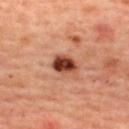Impression:
Captured during whole-body skin photography for melanoma surveillance; the lesion was not biopsied.
Background:
A lesion tile, about 15 mm wide, cut from a 3D total-body photograph. Automated tile analysis of the lesion measured a lesion area of about 6 mm² and a shape-asymmetry score of about 0.2 (0 = symmetric). It also reported a peripheral color-asymmetry measure near 2. The software also gave a classifier nevus-likeness of about 100/100. From the back. This is a cross-polarized tile. The subject is a female aged 43–47.